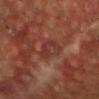Q: Is there a histopathology result?
A: imaged on a skin check; not biopsied
Q: Who is the patient?
A: male, aged around 55
Q: Lesion location?
A: the head or neck
Q: How was this image acquired?
A: ~15 mm crop, total-body skin-cancer survey
Q: What lighting was used for the tile?
A: cross-polarized
Q: Automated lesion metrics?
A: an outline eccentricity of about 0.75 (0 = round, 1 = elongated) and a shape-asymmetry score of about 0.3 (0 = symmetric); an average lesion color of about L≈34 a*≈25 b*≈24 (CIELAB) and a lesion–skin lightness drop of about 5
Q: How large is the lesion?
A: ~3.5 mm (longest diameter)